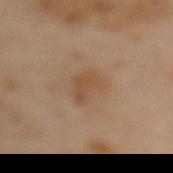workup = imaged on a skin check; not biopsied | image = total-body-photography crop, ~15 mm field of view | subject = male, aged 63–67 | site = the back | diameter = ~3.5 mm (longest diameter).